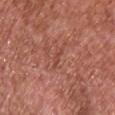location = the back; patient = male, in their mid-60s; acquisition = ~15 mm tile from a whole-body skin photo.